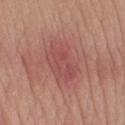Background:
The patient is a male aged 63–67. A roughly 15 mm field-of-view crop from a total-body skin photograph. Located on the right upper arm. About 6 mm across. An algorithmic analysis of the crop reported roughly 7 lightness units darker than nearby skin and a normalized lesion–skin contrast near 5.5. The software also gave an automated nevus-likeness rating near 0 out of 100 and a lesion-detection confidence of about 100/100. Imaged with white-light lighting.